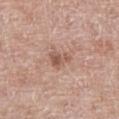Findings:
- follow-up — catalogued during a skin exam; not biopsied
- site — the abdomen
- image-analysis metrics — a mean CIELAB color near L≈55 a*≈21 b*≈27, about 10 CIELAB-L* units darker than the surrounding skin, and a normalized lesion–skin contrast near 7; radial color variation of about 1
- diameter — about 3 mm
- subject — male, aged 68–72
- imaging modality — ~15 mm crop, total-body skin-cancer survey